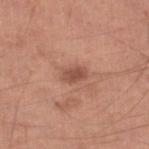From the right lower leg.
A 15 mm close-up extracted from a 3D total-body photography capture.
The patient is a male roughly 65 years of age.